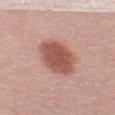Clinical summary:
A close-up tile cropped from a whole-body skin photograph, about 15 mm across. The lesion is located on the left thigh. Approximately 5.5 mm at its widest. The subject is a female in their mid- to late 60s. The total-body-photography lesion software estimated a footprint of about 16 mm² and a symmetry-axis asymmetry near 0.15. It also reported an average lesion color of about L≈54 a*≈26 b*≈26 (CIELAB), a lesion–skin lightness drop of about 14, and a lesion-to-skin contrast of about 9 (normalized; higher = more distinct). The software also gave a border-irregularity index near 1.5/10, a within-lesion color-variation index near 3/10, and peripheral color asymmetry of about 1. The analysis additionally found a classifier nevus-likeness of about 100/100.